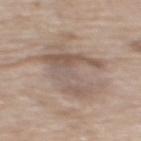Part of a total-body skin-imaging series; this lesion was reviewed on a skin check and was not flagged for biopsy.
The total-body-photography lesion software estimated a footprint of about 18 mm², an outline eccentricity of about 0.6 (0 = round, 1 = elongated), and a symmetry-axis asymmetry near 0.5. And it measured a mean CIELAB color near L≈55 a*≈14 b*≈23, about 8 CIELAB-L* units darker than the surrounding skin, and a lesion-to-skin contrast of about 6 (normalized; higher = more distinct). It also reported a border-irregularity rating of about 9/10, a color-variation rating of about 6/10, and radial color variation of about 1.5. The software also gave an automated nevus-likeness rating near 0 out of 100 and lesion-presence confidence of about 50/100.
Approximately 6 mm at its widest.
Imaged with white-light lighting.
A female subject, aged 73–77.
The lesion is on the upper back.
A close-up tile cropped from a whole-body skin photograph, about 15 mm across.